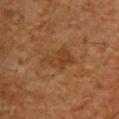Part of a total-body skin-imaging series; this lesion was reviewed on a skin check and was not flagged for biopsy. About 3.5 mm across. This is a cross-polarized tile. A male patient, approximately 65 years of age. From the upper back. The lesion-visualizer software estimated a border-irregularity index near 4.5/10 and a within-lesion color-variation index near 2/10. A 15 mm close-up tile from a total-body photography series done for melanoma screening.